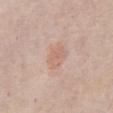Findings:
* site: the abdomen
* patient: male, aged 83 or over
* tile lighting: white-light illumination
* image source: 15 mm crop, total-body photography
* lesion size: ~3.5 mm (longest diameter)